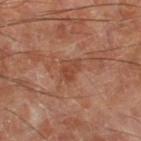Imaged during a routine full-body skin examination; the lesion was not biopsied and no histopathology is available.
Automated image analysis of the tile measured an eccentricity of roughly 0.8 and a shape-asymmetry score of about 0.4 (0 = symmetric). It also reported an average lesion color of about L≈43 a*≈24 b*≈31 (CIELAB), about 7 CIELAB-L* units darker than the surrounding skin, and a normalized lesion–skin contrast near 6. The software also gave a nevus-likeness score of about 0/100 and a lesion-detection confidence of about 100/100.
The patient is a male in their 70s.
The recorded lesion diameter is about 2.5 mm.
The lesion is located on the left thigh.
Imaged with cross-polarized lighting.
A lesion tile, about 15 mm wide, cut from a 3D total-body photograph.